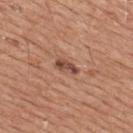biopsy_status: not biopsied; imaged during a skin examination
lighting: white-light
image:
  source: total-body photography crop
  field_of_view_mm: 15
lesion_size:
  long_diameter_mm_approx: 3.0
site: upper back
patient:
  sex: male
  age_approx: 65